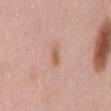<lesion>
  <biopsy_status>not biopsied; imaged during a skin examination</biopsy_status>
  <lighting>white-light</lighting>
  <automated_metrics>
    <area_mm2_approx>3.0</area_mm2_approx>
    <shape_asymmetry>0.2</shape_asymmetry>
    <lesion_detection_confidence_0_100>100</lesion_detection_confidence_0_100>
  </automated_metrics>
  <image>
    <source>total-body photography crop</source>
    <field_of_view_mm>15</field_of_view_mm>
  </image>
  <patient>
    <sex>female</sex>
    <age_approx>50</age_approx>
  </patient>
  <site>mid back</site>
  <lesion_size>
    <long_diameter_mm_approx>2.5</long_diameter_mm_approx>
  </lesion_size>
</lesion>